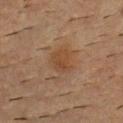Assessment:
Part of a total-body skin-imaging series; this lesion was reviewed on a skin check and was not flagged for biopsy.
Background:
The subject is a male roughly 55 years of age. A 15 mm crop from a total-body photograph taken for skin-cancer surveillance. The total-body-photography lesion software estimated a footprint of about 6 mm², an outline eccentricity of about 0.6 (0 = round, 1 = elongated), and a symmetry-axis asymmetry near 0.3. The software also gave a nevus-likeness score of about 5/100 and a lesion-detection confidence of about 100/100. Located on the upper back.